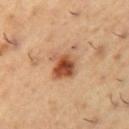biopsy status: imaged on a skin check; not biopsied
patient: male, aged 53 to 57
illumination: cross-polarized
lesion diameter: ~4 mm (longest diameter)
image-analysis metrics: border irregularity of about 3.5 on a 0–10 scale and radial color variation of about 3; an automated nevus-likeness rating near 95 out of 100
location: the left upper arm
acquisition: ~15 mm crop, total-body skin-cancer survey